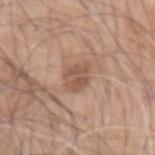biopsy status: catalogued during a skin exam; not biopsied | imaging modality: ~15 mm crop, total-body skin-cancer survey | tile lighting: white-light illumination | patient: male, in their 60s | lesion diameter: ≈3 mm | body site: the left upper arm.